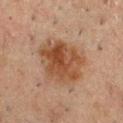workup: no biopsy performed (imaged during a skin exam)
anatomic site: the front of the torso
patient: male, aged 48–52
diameter: about 6.5 mm
acquisition: 15 mm crop, total-body photography
automated metrics: an average lesion color of about L≈37 a*≈17 b*≈27 (CIELAB) and roughly 9 lightness units darker than nearby skin; a within-lesion color-variation index near 5.5/10 and a peripheral color-asymmetry measure near 2; a detector confidence of about 100 out of 100 that the crop contains a lesion